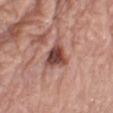Clinical impression:
The lesion was photographed on a routine skin check and not biopsied; there is no pathology result.
Context:
A male patient aged approximately 80. A lesion tile, about 15 mm wide, cut from a 3D total-body photograph. From the leg. The tile uses white-light illumination. Automated image analysis of the tile measured a footprint of about 6.5 mm² and an outline eccentricity of about 0.55 (0 = round, 1 = elongated). The software also gave an average lesion color of about L≈45 a*≈24 b*≈25 (CIELAB), about 16 CIELAB-L* units darker than the surrounding skin, and a normalized lesion–skin contrast near 11. The software also gave a nevus-likeness score of about 45/100 and a detector confidence of about 100 out of 100 that the crop contains a lesion.